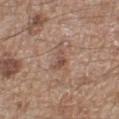Captured during whole-body skin photography for melanoma surveillance; the lesion was not biopsied. A lesion tile, about 15 mm wide, cut from a 3D total-body photograph. This is a white-light tile. Approximately 3.5 mm at its widest. A male patient, aged 63–67. On the left lower leg. The total-body-photography lesion software estimated a lesion area of about 4.5 mm² and a shape-asymmetry score of about 0.6 (0 = symmetric). The analysis additionally found a border-irregularity index near 6/10, a color-variation rating of about 4/10, and radial color variation of about 1.5. It also reported an automated nevus-likeness rating near 0 out of 100 and lesion-presence confidence of about 100/100.